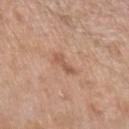Q: Who is the patient?
A: male, aged approximately 65
Q: Illumination type?
A: white-light illumination
Q: What is the anatomic site?
A: the left upper arm
Q: How large is the lesion?
A: about 2.5 mm
Q: What did automated image analysis measure?
A: an area of roughly 3 mm², an outline eccentricity of about 0.9 (0 = round, 1 = elongated), and two-axis asymmetry of about 0.4; a lesion color around L≈55 a*≈21 b*≈31 in CIELAB, roughly 9 lightness units darker than nearby skin, and a lesion-to-skin contrast of about 6.5 (normalized; higher = more distinct)
Q: What kind of image is this?
A: total-body-photography crop, ~15 mm field of view